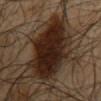Captured during whole-body skin photography for melanoma surveillance; the lesion was not biopsied. A male subject about 65 years old. The tile uses cross-polarized illumination. From the back. A close-up tile cropped from a whole-body skin photograph, about 15 mm across. An algorithmic analysis of the crop reported a lesion-detection confidence of about 65/100.